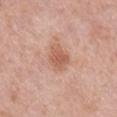workup — catalogued during a skin exam; not biopsied | lesion size — ~3 mm (longest diameter) | subject — female, approximately 50 years of age | imaging modality — 15 mm crop, total-body photography | tile lighting — white-light | location — the right lower leg.